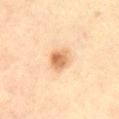follow-up = total-body-photography surveillance lesion; no biopsy
lesion diameter = ~2.5 mm (longest diameter)
acquisition = ~15 mm crop, total-body skin-cancer survey
subject = female, approximately 60 years of age
site = the abdomen